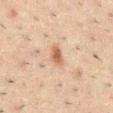This lesion was catalogued during total-body skin photography and was not selected for biopsy.